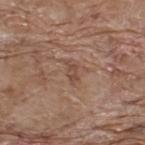Captured during whole-body skin photography for melanoma surveillance; the lesion was not biopsied.
A male patient aged 68–72.
A 15 mm crop from a total-body photograph taken for skin-cancer surveillance.
On the back.